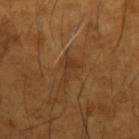biopsy_status: not biopsied; imaged during a skin examination
site: left forearm
patient:
  sex: male
  age_approx: 65
image:
  source: total-body photography crop
  field_of_view_mm: 15